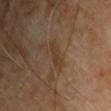Case summary:
* follow-up — imaged on a skin check; not biopsied
* image source — 15 mm crop, total-body photography
* subject — male, in their 70s
* tile lighting — cross-polarized illumination
* diameter — ≈3.5 mm
* body site — the chest
* automated lesion analysis — two-axis asymmetry of about 0.3; an average lesion color of about L≈36 a*≈16 b*≈28 (CIELAB), roughly 6 lightness units darker than nearby skin, and a normalized lesion–skin contrast near 6.5